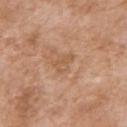Imaged during a routine full-body skin examination; the lesion was not biopsied and no histopathology is available. This image is a 15 mm lesion crop taken from a total-body photograph. An algorithmic analysis of the crop reported a lesion area of about 3.5 mm², an eccentricity of roughly 0.75, and a shape-asymmetry score of about 0.45 (0 = symmetric). And it measured a mean CIELAB color near L≈56 a*≈20 b*≈34 and a normalized lesion–skin contrast near 5. And it measured a border-irregularity index near 4.5/10, internal color variation of about 1.5 on a 0–10 scale, and peripheral color asymmetry of about 0.5. The subject is a female about 75 years old. This is a white-light tile. The lesion is on the right upper arm. The recorded lesion diameter is about 2.5 mm.